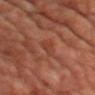Clinical impression: No biopsy was performed on this lesion — it was imaged during a full skin examination and was not determined to be concerning. Clinical summary: From the chest. A close-up tile cropped from a whole-body skin photograph, about 15 mm across. Automated tile analysis of the lesion measured an outline eccentricity of about 0.8 (0 = round, 1 = elongated) and two-axis asymmetry of about 0.35. It also reported a classifier nevus-likeness of about 0/100 and a detector confidence of about 95 out of 100 that the crop contains a lesion. A male subject about 55 years old. Longest diameter approximately 2.5 mm.